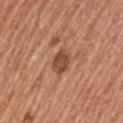Captured during whole-body skin photography for melanoma surveillance; the lesion was not biopsied. About 3 mm across. This is a white-light tile. A male patient, aged 53 to 57. The lesion is located on the left upper arm. A close-up tile cropped from a whole-body skin photograph, about 15 mm across.